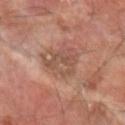Clinical impression: Part of a total-body skin-imaging series; this lesion was reviewed on a skin check and was not flagged for biopsy. Acquisition and patient details: The lesion is located on the left forearm. A lesion tile, about 15 mm wide, cut from a 3D total-body photograph. A male patient approximately 65 years of age.